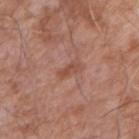anatomic site: the arm; patient: male, aged 73–77; image source: ~15 mm tile from a whole-body skin photo.